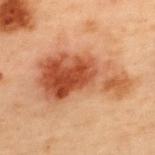follow-up: total-body-photography surveillance lesion; no biopsy
acquisition: 15 mm crop, total-body photography
body site: the upper back
patient: male, aged 53–57